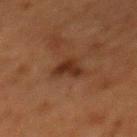Impression:
No biopsy was performed on this lesion — it was imaged during a full skin examination and was not determined to be concerning.
Background:
On the back. Measured at roughly 3 mm in maximum diameter. A female subject approximately 50 years of age. This is a cross-polarized tile. A roughly 15 mm field-of-view crop from a total-body skin photograph. The lesion-visualizer software estimated a border-irregularity index near 4/10, a within-lesion color-variation index near 2.5/10, and a peripheral color-asymmetry measure near 1.